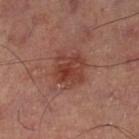Recorded during total-body skin imaging; not selected for excision or biopsy. On the left thigh. About 4 mm across. An algorithmic analysis of the crop reported a color-variation rating of about 5/10 and a peripheral color-asymmetry measure near 1.5. And it measured an automated nevus-likeness rating near 60 out of 100 and lesion-presence confidence of about 100/100. This is a cross-polarized tile. A lesion tile, about 15 mm wide, cut from a 3D total-body photograph.